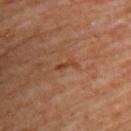This lesion was catalogued during total-body skin photography and was not selected for biopsy.
A 15 mm close-up extracted from a 3D total-body photography capture.
Approximately 2.5 mm at its widest.
Imaged with cross-polarized lighting.
From the upper back.
The subject is a male about 70 years old.
The lesion-visualizer software estimated a lesion color around L≈41 a*≈24 b*≈33 in CIELAB, roughly 7 lightness units darker than nearby skin, and a lesion-to-skin contrast of about 6.5 (normalized; higher = more distinct). It also reported a classifier nevus-likeness of about 0/100 and a detector confidence of about 95 out of 100 that the crop contains a lesion.